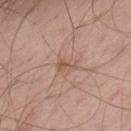Q: Is there a histopathology result?
A: catalogued during a skin exam; not biopsied
Q: Where on the body is the lesion?
A: the upper back
Q: How large is the lesion?
A: ~2.5 mm (longest diameter)
Q: Patient demographics?
A: male, approximately 65 years of age
Q: What is the imaging modality?
A: total-body-photography crop, ~15 mm field of view
Q: What did automated image analysis measure?
A: a lesion area of about 3 mm², an outline eccentricity of about 0.85 (0 = round, 1 = elongated), and two-axis asymmetry of about 0.55; a lesion color around L≈55 a*≈18 b*≈28 in CIELAB, roughly 8 lightness units darker than nearby skin, and a normalized lesion–skin contrast near 6